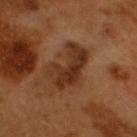Q: Is there a histopathology result?
A: catalogued during a skin exam; not biopsied
Q: Lesion location?
A: the head or neck
Q: What is the imaging modality?
A: total-body-photography crop, ~15 mm field of view
Q: Who is the patient?
A: male, roughly 60 years of age